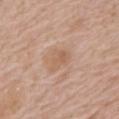Imaged during a routine full-body skin examination; the lesion was not biopsied and no histopathology is available. From the mid back. Automated image analysis of the tile measured a lesion area of about 6.5 mm², an outline eccentricity of about 0.75 (0 = round, 1 = elongated), and a shape-asymmetry score of about 0.3 (0 = symmetric). Approximately 3.5 mm at its widest. Cropped from a total-body skin-imaging series; the visible field is about 15 mm. A female subject aged 73–77.